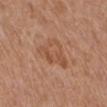| field | value |
|---|---|
| notes | no biopsy performed (imaged during a skin exam) |
| imaging modality | ~15 mm tile from a whole-body skin photo |
| patient | female, aged 53 to 57 |
| lighting | white-light illumination |
| diameter | ~5 mm (longest diameter) |
| site | the right lower leg |